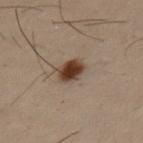| field | value |
|---|---|
| biopsy status | catalogued during a skin exam; not biopsied |
| body site | the left upper arm |
| illumination | cross-polarized illumination |
| diameter | about 3 mm |
| subject | male, aged 28–32 |
| image | ~15 mm tile from a whole-body skin photo |
| automated lesion analysis | a lesion color around L≈31 a*≈14 b*≈22 in CIELAB and a normalized border contrast of about 12.5 |